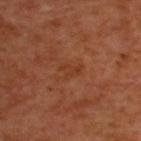Findings:
- biopsy status: no biopsy performed (imaged during a skin exam)
- imaging modality: ~15 mm tile from a whole-body skin photo
- site: the upper back
- subject: male, approximately 50 years of age
- lesion diameter: ~2.5 mm (longest diameter)
- tile lighting: cross-polarized illumination